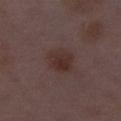Q: What did automated image analysis measure?
A: a lesion color around L≈30 a*≈17 b*≈18 in CIELAB, a lesion–skin lightness drop of about 6, and a lesion-to-skin contrast of about 7 (normalized; higher = more distinct); a classifier nevus-likeness of about 85/100 and a detector confidence of about 100 out of 100 that the crop contains a lesion
Q: How large is the lesion?
A: ≈4 mm
Q: Patient demographics?
A: female, aged 28 to 32
Q: How was this image acquired?
A: total-body-photography crop, ~15 mm field of view
Q: Where on the body is the lesion?
A: the right thigh